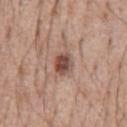Recorded during total-body skin imaging; not selected for excision or biopsy. A male subject aged approximately 55. A 15 mm crop from a total-body photograph taken for skin-cancer surveillance. On the front of the torso.